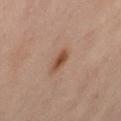Recorded during total-body skin imaging; not selected for excision or biopsy.
The lesion is on the abdomen.
A close-up tile cropped from a whole-body skin photograph, about 15 mm across.
The patient is a female aged 53 to 57.